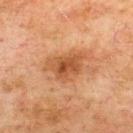Assessment: Part of a total-body skin-imaging series; this lesion was reviewed on a skin check and was not flagged for biopsy. Image and clinical context: The tile uses cross-polarized illumination. From the upper back. A 15 mm close-up tile from a total-body photography series done for melanoma screening. A male patient aged 73 to 77.